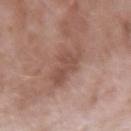workup=catalogued during a skin exam; not biopsied | body site=the left forearm | image=~15 mm tile from a whole-body skin photo | lighting=white-light | size=about 4.5 mm | subject=female, roughly 60 years of age.